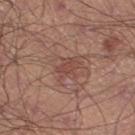Captured during whole-body skin photography for melanoma surveillance; the lesion was not biopsied. The recorded lesion diameter is about 3 mm. The lesion is on the right thigh. Captured under white-light illumination. A close-up tile cropped from a whole-body skin photograph, about 15 mm across. Automated tile analysis of the lesion measured an average lesion color of about L≈46 a*≈22 b*≈25 (CIELAB), roughly 7 lightness units darker than nearby skin, and a lesion-to-skin contrast of about 5 (normalized; higher = more distinct). The analysis additionally found a border-irregularity rating of about 2.5/10, internal color variation of about 1.5 on a 0–10 scale, and radial color variation of about 0.5. The analysis additionally found an automated nevus-likeness rating near 0 out of 100 and a detector confidence of about 100 out of 100 that the crop contains a lesion. A male patient, about 45 years old.